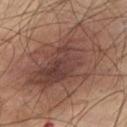Q: Is there a histopathology result?
A: imaged on a skin check; not biopsied
Q: What are the patient's age and sex?
A: male, aged 53–57
Q: What is the lesion's diameter?
A: ≈11 mm
Q: What is the anatomic site?
A: the right thigh
Q: Automated lesion metrics?
A: a lesion area of about 60 mm² and a shape-asymmetry score of about 0.2 (0 = symmetric); an average lesion color of about L≈39 a*≈17 b*≈22 (CIELAB) and a lesion-to-skin contrast of about 8.5 (normalized; higher = more distinct); a border-irregularity index near 3/10, a within-lesion color-variation index near 6.5/10, and a peripheral color-asymmetry measure near 2.5
Q: What is the imaging modality?
A: ~15 mm crop, total-body skin-cancer survey
Q: How was the tile lit?
A: cross-polarized illumination